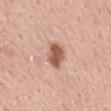Q: Was a biopsy performed?
A: no biopsy performed (imaged during a skin exam)
Q: How was this image acquired?
A: total-body-photography crop, ~15 mm field of view
Q: What did automated image analysis measure?
A: a footprint of about 7 mm²; a mean CIELAB color near L≈57 a*≈23 b*≈30, a lesion–skin lightness drop of about 14, and a normalized lesion–skin contrast near 9.5; a classifier nevus-likeness of about 85/100 and a detector confidence of about 100 out of 100 that the crop contains a lesion
Q: What is the anatomic site?
A: the mid back
Q: What are the patient's age and sex?
A: male, approximately 55 years of age
Q: How was the tile lit?
A: white-light illumination
Q: Lesion size?
A: ~3 mm (longest diameter)